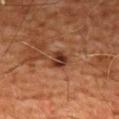Assessment:
Captured during whole-body skin photography for melanoma surveillance; the lesion was not biopsied.
Acquisition and patient details:
A male subject aged around 60. Imaged with cross-polarized lighting. From the chest. This image is a 15 mm lesion crop taken from a total-body photograph. An algorithmic analysis of the crop reported a shape-asymmetry score of about 0.2 (0 = symmetric). It also reported lesion-presence confidence of about 100/100.